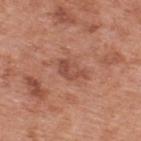Impression:
Recorded during total-body skin imaging; not selected for excision or biopsy.
Context:
Imaged with white-light lighting. Longest diameter approximately 3.5 mm. A roughly 15 mm field-of-view crop from a total-body skin photograph. The lesion is on the back. A male patient in their 70s.